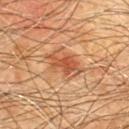workup: total-body-photography surveillance lesion; no biopsy
patient: male, approximately 60 years of age
tile lighting: cross-polarized illumination
body site: the chest
image-analysis metrics: a mean CIELAB color near L≈49 a*≈26 b*≈36, about 10 CIELAB-L* units darker than the surrounding skin, and a normalized lesion–skin contrast near 7.5; a border-irregularity index near 5/10, internal color variation of about 3.5 on a 0–10 scale, and a peripheral color-asymmetry measure near 1.5
lesion size: about 4.5 mm
acquisition: ~15 mm tile from a whole-body skin photo